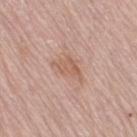Q: Was this lesion biopsied?
A: imaged on a skin check; not biopsied
Q: Automated lesion metrics?
A: a lesion color around L≈59 a*≈20 b*≈29 in CIELAB, about 8 CIELAB-L* units darker than the surrounding skin, and a normalized lesion–skin contrast near 6; a border-irregularity rating of about 5/10 and a color-variation rating of about 2/10; a nevus-likeness score of about 0/100 and lesion-presence confidence of about 100/100
Q: Illumination type?
A: white-light illumination
Q: How was this image acquired?
A: total-body-photography crop, ~15 mm field of view
Q: What are the patient's age and sex?
A: female, aged around 65
Q: Lesion size?
A: about 4 mm
Q: Lesion location?
A: the left leg